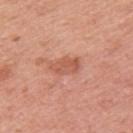Assessment: No biopsy was performed on this lesion — it was imaged during a full skin examination and was not determined to be concerning. Context: A female subject, aged 48–52. From the left upper arm. This is a white-light tile. Longest diameter approximately 3.5 mm. Cropped from a whole-body photographic skin survey; the tile spans about 15 mm.